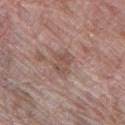This lesion was catalogued during total-body skin photography and was not selected for biopsy.
The recorded lesion diameter is about 3 mm.
A roughly 15 mm field-of-view crop from a total-body skin photograph.
A female patient, about 70 years old.
The lesion is on the left thigh.
Imaged with white-light lighting.
An algorithmic analysis of the crop reported a footprint of about 5.5 mm², an outline eccentricity of about 0.7 (0 = round, 1 = elongated), and two-axis asymmetry of about 0.35. It also reported an average lesion color of about L≈51 a*≈19 b*≈23 (CIELAB), a lesion–skin lightness drop of about 8, and a normalized border contrast of about 5.5. It also reported a border-irregularity rating of about 3.5/10, a within-lesion color-variation index near 3.5/10, and radial color variation of about 1. The software also gave an automated nevus-likeness rating near 0 out of 100 and lesion-presence confidence of about 95/100.